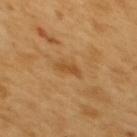  biopsy_status: not biopsied; imaged during a skin examination
  patient:
    sex: female
    age_approx: 55
  image:
    source: total-body photography crop
    field_of_view_mm: 15
  lighting: cross-polarized
  lesion_size:
    long_diameter_mm_approx: 2.5
  site: upper back
  automated_metrics:
    area_mm2_approx: 3.0
    shape_asymmetry: 0.35
    cielab_L: 50
    cielab_a: 22
    cielab_b: 42
    vs_skin_darker_L: 8.0
    vs_skin_contrast_norm: 6.0
    nevus_likeness_0_100: 0
    lesion_detection_confidence_0_100: 100